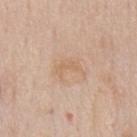| feature | finding |
|---|---|
| image source | ~15 mm crop, total-body skin-cancer survey |
| location | the front of the torso |
| patient | male, aged 58–62 |
| automated metrics | a lesion area of about 5 mm² and an outline eccentricity of about 0.8 (0 = round, 1 = elongated) |
| lesion size | about 3.5 mm |